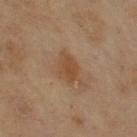No biopsy was performed on this lesion — it was imaged during a full skin examination and was not determined to be concerning.
The lesion is located on the arm.
The subject is a female in their mid-60s.
A 15 mm close-up tile from a total-body photography series done for melanoma screening.